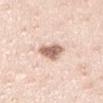Clinical summary:
A region of skin cropped from a whole-body photographic capture, roughly 15 mm wide. A male subject, aged approximately 35. Located on the right upper arm. Captured under white-light illumination.